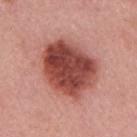Findings:
- workup · total-body-photography surveillance lesion; no biopsy
- automated metrics · an average lesion color of about L≈47 a*≈29 b*≈27 (CIELAB), about 18 CIELAB-L* units darker than the surrounding skin, and a normalized border contrast of about 12; a border-irregularity index near 1.5/10, a color-variation rating of about 7/10, and peripheral color asymmetry of about 2.5
- size · ≈7.5 mm
- acquisition · 15 mm crop, total-body photography
- tile lighting · white-light illumination
- site · the mid back
- subject · male, aged 58 to 62